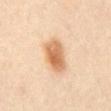| field | value |
|---|---|
| biopsy status | imaged on a skin check; not biopsied |
| patient | male, roughly 45 years of age |
| imaging modality | ~15 mm crop, total-body skin-cancer survey |
| site | the abdomen |